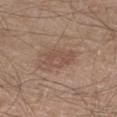notes: no biopsy performed (imaged during a skin exam) | diameter: about 4 mm | acquisition: ~15 mm tile from a whole-body skin photo | anatomic site: the right lower leg | lighting: white-light illumination | automated lesion analysis: an area of roughly 6.5 mm², a shape eccentricity near 0.75, and two-axis asymmetry of about 0.25; a mean CIELAB color near L≈50 a*≈18 b*≈25 and roughly 7 lightness units darker than nearby skin | patient: male, roughly 60 years of age.